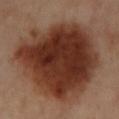Part of a total-body skin-imaging series; this lesion was reviewed on a skin check and was not flagged for biopsy. A female subject, about 55 years old. Automated tile analysis of the lesion measured a mean CIELAB color near L≈35 a*≈22 b*≈28, about 16 CIELAB-L* units darker than the surrounding skin, and a lesion-to-skin contrast of about 13 (normalized; higher = more distinct). The software also gave a classifier nevus-likeness of about 100/100 and lesion-presence confidence of about 100/100. A lesion tile, about 15 mm wide, cut from a 3D total-body photograph. The lesion is located on the left lower leg. Imaged with cross-polarized lighting.